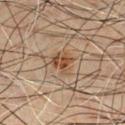This lesion was catalogued during total-body skin photography and was not selected for biopsy.
The lesion-visualizer software estimated an automated nevus-likeness rating near 75 out of 100 and a detector confidence of about 100 out of 100 that the crop contains a lesion.
The lesion is located on the chest.
A male patient about 60 years old.
Captured under cross-polarized illumination.
A close-up tile cropped from a whole-body skin photograph, about 15 mm across.
The lesion's longest dimension is about 2.5 mm.